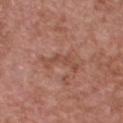workup: no biopsy performed (imaged during a skin exam) | lesion size: about 4.5 mm | tile lighting: white-light | site: the chest | automated lesion analysis: an outline eccentricity of about 0.9 (0 = round, 1 = elongated) and two-axis asymmetry of about 0.7; a lesion–skin lightness drop of about 7 and a normalized lesion–skin contrast near 5.5; a border-irregularity rating of about 9/10, a within-lesion color-variation index near 0.5/10, and a peripheral color-asymmetry measure near 0; a nevus-likeness score of about 0/100 and lesion-presence confidence of about 100/100 | subject: male, aged 63 to 67 | acquisition: ~15 mm crop, total-body skin-cancer survey.